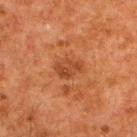Background: Approximately 3.5 mm at its widest. A male subject, roughly 60 years of age. A close-up tile cropped from a whole-body skin photograph, about 15 mm across. Located on the upper back. The total-body-photography lesion software estimated a footprint of about 6.5 mm². It also reported a border-irregularity index near 2/10, a color-variation rating of about 2.5/10, and peripheral color asymmetry of about 1. The software also gave an automated nevus-likeness rating near 0 out of 100 and a detector confidence of about 100 out of 100 that the crop contains a lesion. Imaged with cross-polarized lighting.